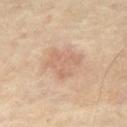| key | value |
|---|---|
| notes | catalogued during a skin exam; not biopsied |